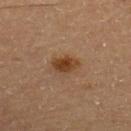follow-up: no biopsy performed (imaged during a skin exam) | subject: male, in their 60s | lesion diameter: ≈3 mm | image: ~15 mm crop, total-body skin-cancer survey | image-analysis metrics: roughly 9 lightness units darker than nearby skin; lesion-presence confidence of about 100/100.